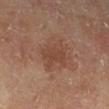Q: Was this lesion biopsied?
A: no biopsy performed (imaged during a skin exam)
Q: What are the patient's age and sex?
A: male, approximately 65 years of age
Q: Illumination type?
A: cross-polarized
Q: What is the imaging modality?
A: ~15 mm tile from a whole-body skin photo
Q: How large is the lesion?
A: about 3.5 mm
Q: Where on the body is the lesion?
A: the right lower leg